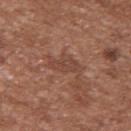Findings:
- follow-up: total-body-photography surveillance lesion; no biopsy